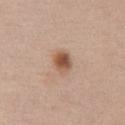biopsy status=catalogued during a skin exam; not biopsied | image=~15 mm tile from a whole-body skin photo | patient=female, about 40 years old | location=the front of the torso | lesion size=~3 mm (longest diameter) | image-analysis metrics=border irregularity of about 1.5 on a 0–10 scale, a color-variation rating of about 5/10, and a peripheral color-asymmetry measure near 1.5; a classifier nevus-likeness of about 95/100 and lesion-presence confidence of about 100/100.